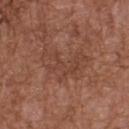Case summary:
– follow-up: total-body-photography surveillance lesion; no biopsy
– patient: male, in their mid- to late 70s
– image source: ~15 mm tile from a whole-body skin photo
– location: the upper back
– lighting: white-light
– size: about 6 mm